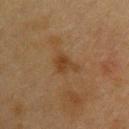Clinical impression:
No biopsy was performed on this lesion — it was imaged during a full skin examination and was not determined to be concerning.
Acquisition and patient details:
A female patient, in their 40s. A lesion tile, about 15 mm wide, cut from a 3D total-body photograph. The tile uses cross-polarized illumination. From the upper back.